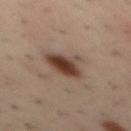Recorded during total-body skin imaging; not selected for excision or biopsy.
The lesion-visualizer software estimated a lesion area of about 9.5 mm² and a shape-asymmetry score of about 0.25 (0 = symmetric). The analysis additionally found a border-irregularity rating of about 3/10, internal color variation of about 5.5 on a 0–10 scale, and a peripheral color-asymmetry measure near 1.5.
Approximately 4.5 mm at its widest.
The lesion is on the mid back.
Imaged with cross-polarized lighting.
The subject is a male in their 40s.
A 15 mm close-up tile from a total-body photography series done for melanoma screening.